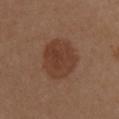| key | value |
|---|---|
| follow-up | total-body-photography surveillance lesion; no biopsy |
| anatomic site | the arm |
| acquisition | ~15 mm tile from a whole-body skin photo |
| subject | female, approximately 40 years of age |
| automated metrics | a footprint of about 17 mm², an outline eccentricity of about 0.6 (0 = round, 1 = elongated), and a symmetry-axis asymmetry near 0.2; a lesion color around L≈38 a*≈20 b*≈28 in CIELAB, a lesion–skin lightness drop of about 8, and a lesion-to-skin contrast of about 7.5 (normalized; higher = more distinct); a border-irregularity rating of about 2/10 and a color-variation rating of about 3/10 |
| lesion diameter | ≈5 mm |